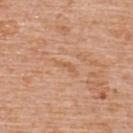The lesion was tiled from a total-body skin photograph and was not biopsied. The subject is a female approximately 55 years of age. The lesion is located on the upper back. A lesion tile, about 15 mm wide, cut from a 3D total-body photograph. Captured under white-light illumination.